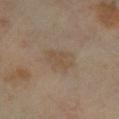No biopsy was performed on this lesion — it was imaged during a full skin examination and was not determined to be concerning. Cropped from a whole-body photographic skin survey; the tile spans about 15 mm. A male subject in their mid- to late 60s. Approximately 4 mm at its widest. On the left lower leg. Imaged with cross-polarized lighting.